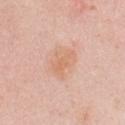Clinical impression: Recorded during total-body skin imaging; not selected for excision or biopsy. Clinical summary: On the chest. A female patient aged 28–32. A close-up tile cropped from a whole-body skin photograph, about 15 mm across. About 4 mm across. This is a white-light tile.